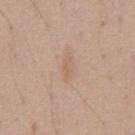Case summary:
* notes · total-body-photography surveillance lesion; no biopsy
* image · ~15 mm crop, total-body skin-cancer survey
* illumination · white-light illumination
* patient · male, aged 38 to 42
* body site · the abdomen
* size · about 3 mm